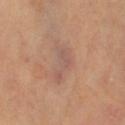| feature | finding |
|---|---|
| workup | catalogued during a skin exam; not biopsied |
| patient | female, in their 70s |
| acquisition | ~15 mm tile from a whole-body skin photo |
| location | the right thigh |
| diameter | ~4.5 mm (longest diameter) |